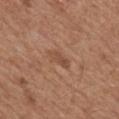Imaged during a routine full-body skin examination; the lesion was not biopsied and no histopathology is available. The lesion is located on the mid back. Cropped from a whole-body photographic skin survey; the tile spans about 15 mm. The tile uses white-light illumination. Approximately 3 mm at its widest. A male subject, in their mid-60s.